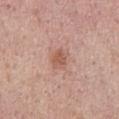Impression:
The lesion was tiled from a total-body skin photograph and was not biopsied.
Acquisition and patient details:
The lesion is located on the mid back. A roughly 15 mm field-of-view crop from a total-body skin photograph. A male patient about 55 years old. Captured under white-light illumination. Measured at roughly 2.5 mm in maximum diameter.